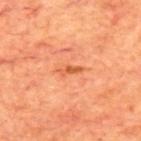<case>
  <biopsy_status>not biopsied; imaged during a skin examination</biopsy_status>
  <image>
    <source>total-body photography crop</source>
    <field_of_view_mm>15</field_of_view_mm>
  </image>
  <patient>
    <sex>male</sex>
    <age_approx>70</age_approx>
  </patient>
  <site>upper back</site>
  <automated_metrics>
    <shape_asymmetry>0.5</shape_asymmetry>
    <border_irregularity_0_10>5.0</border_irregularity_0_10>
    <color_variation_0_10>0.0</color_variation_0_10>
    <peripheral_color_asymmetry>0.0</peripheral_color_asymmetry>
  </automated_metrics>
  <lesion_size>
    <long_diameter_mm_approx>3.0</long_diameter_mm_approx>
  </lesion_size>
</case>